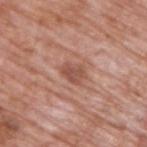  biopsy_status: not biopsied; imaged during a skin examination
  site: back
  image:
    source: total-body photography crop
    field_of_view_mm: 15
  automated_metrics:
    border_irregularity_0_10: 2.5
    peripheral_color_asymmetry: 1.0
    nevus_likeness_0_100: 15
    lesion_detection_confidence_0_100: 100
  patient:
    sex: male
    age_approx: 70
  lesion_size:
    long_diameter_mm_approx: 3.0
  lighting: white-light